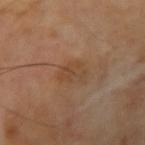• biopsy status: imaged on a skin check; not biopsied
• image-analysis metrics: a lesion area of about 5 mm², an eccentricity of roughly 0.8, and two-axis asymmetry of about 0.3; a lesion color around L≈43 a*≈18 b*≈31 in CIELAB and a normalized border contrast of about 5.5; a classifier nevus-likeness of about 0/100 and a detector confidence of about 100 out of 100 that the crop contains a lesion
• subject: male, aged 68–72
• body site: the arm
• imaging modality: total-body-photography crop, ~15 mm field of view
• lesion size: ≈3 mm